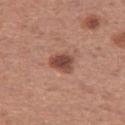Impression: Part of a total-body skin-imaging series; this lesion was reviewed on a skin check and was not flagged for biopsy. Image and clinical context: Measured at roughly 3 mm in maximum diameter. A 15 mm close-up extracted from a 3D total-body photography capture. The lesion is located on the right thigh. This is a white-light tile. A female subject roughly 30 years of age.